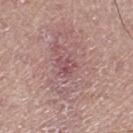On the leg. Cropped from a whole-body photographic skin survey; the tile spans about 15 mm. The patient is a male in their mid-60s. An algorithmic analysis of the crop reported an eccentricity of roughly 0.6 and a shape-asymmetry score of about 0.35 (0 = symmetric).